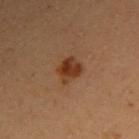Case summary:
• notes — total-body-photography surveillance lesion; no biopsy
• lighting — cross-polarized illumination
• patient — female, in their 40s
• image — ~15 mm crop, total-body skin-cancer survey
• lesion diameter — ~3 mm (longest diameter)
• automated lesion analysis — a lesion area of about 5.5 mm², an eccentricity of roughly 0.55, and a shape-asymmetry score of about 0.2 (0 = symmetric); a border-irregularity index near 2.5/10, internal color variation of about 4 on a 0–10 scale, and peripheral color asymmetry of about 1.5; an automated nevus-likeness rating near 95 out of 100 and lesion-presence confidence of about 100/100
• site — the right upper arm